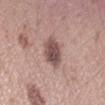  biopsy_status: not biopsied; imaged during a skin examination
  lesion_size:
    long_diameter_mm_approx: 4.0
  site: arm
  image:
    source: total-body photography crop
    field_of_view_mm: 15
  patient:
    sex: female
    age_approx: 35
  lighting: white-light
  automated_metrics:
    color_variation_0_10: 4.0
    peripheral_color_asymmetry: 1.0
    lesion_detection_confidence_0_100: 100